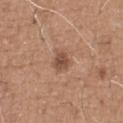follow-up = catalogued during a skin exam; not biopsied | location = the chest | tile lighting = white-light illumination | diameter = about 2.5 mm | image-analysis metrics = an eccentricity of roughly 0.65 and a symmetry-axis asymmetry near 0.25; a lesion color around L≈49 a*≈21 b*≈28 in CIELAB, roughly 11 lightness units darker than nearby skin, and a normalized border contrast of about 8; a border-irregularity rating of about 2/10, a color-variation rating of about 2.5/10, and peripheral color asymmetry of about 1 | imaging modality = ~15 mm crop, total-body skin-cancer survey | patient = male, aged around 65.